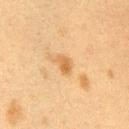Impression:
The lesion was tiled from a total-body skin photograph and was not biopsied.
Context:
From the right upper arm. The tile uses cross-polarized illumination. The patient is a female aged approximately 40. The total-body-photography lesion software estimated an area of roughly 3.5 mm² and a symmetry-axis asymmetry near 0.3. And it measured a border-irregularity rating of about 3/10, a within-lesion color-variation index near 2/10, and peripheral color asymmetry of about 0.5. The analysis additionally found a lesion-detection confidence of about 100/100. A close-up tile cropped from a whole-body skin photograph, about 15 mm across.